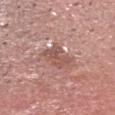<tbp_lesion>
  <automated_metrics>
    <area_mm2_approx>6.5</area_mm2_approx>
    <eccentricity>0.35</eccentricity>
    <shape_asymmetry>0.25</shape_asymmetry>
    <cielab_L>53</cielab_L>
    <cielab_a>22</cielab_a>
    <cielab_b>25</cielab_b>
    <vs_skin_contrast_norm>6.5</vs_skin_contrast_norm>
    <lesion_detection_confidence_0_100>65</lesion_detection_confidence_0_100>
  </automated_metrics>
  <site>head or neck</site>
  <lesion_size>
    <long_diameter_mm_approx>3.0</long_diameter_mm_approx>
  </lesion_size>
  <image>
    <source>total-body photography crop</source>
    <field_of_view_mm>15</field_of_view_mm>
  </image>
  <patient>
    <sex>male</sex>
    <age_approx>55</age_approx>
  </patient>
  <lighting>white-light</lighting>
</tbp_lesion>